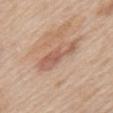workup: imaged on a skin check; not biopsied
location: the upper back
subject: male, approximately 60 years of age
automated metrics: a lesion area of about 9 mm², an eccentricity of roughly 0.95, and a symmetry-axis asymmetry near 0.45; a lesion color around L≈58 a*≈21 b*≈29 in CIELAB, about 9 CIELAB-L* units darker than the surrounding skin, and a normalized lesion–skin contrast near 6.5; a color-variation rating of about 2.5/10 and a peripheral color-asymmetry measure near 1
imaging modality: ~15 mm tile from a whole-body skin photo
size: ≈5.5 mm
tile lighting: white-light illumination